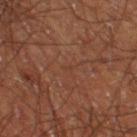Assessment: The lesion was photographed on a routine skin check and not biopsied; there is no pathology result. Clinical summary: The lesion is on the leg. A lesion tile, about 15 mm wide, cut from a 3D total-body photograph. A male subject aged 58–62. Imaged with cross-polarized lighting. An algorithmic analysis of the crop reported a footprint of about 1 mm², an outline eccentricity of about 0.85 (0 = round, 1 = elongated), and a shape-asymmetry score of about 0.55 (0 = symmetric). It also reported a lesion color around L≈34 a*≈20 b*≈26 in CIELAB, roughly 3 lightness units darker than nearby skin, and a normalized border contrast of about 3. Measured at roughly 1 mm in maximum diameter.